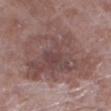Notes:
- workup: total-body-photography surveillance lesion; no biopsy
- image: ~15 mm tile from a whole-body skin photo
- illumination: white-light
- patient: female, aged 68 to 72
- automated metrics: a lesion area of about 60 mm² and a shape-asymmetry score of about 0.35 (0 = symmetric)
- lesion diameter: ≈10.5 mm
- site: the left lower leg